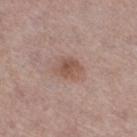Impression: Captured during whole-body skin photography for melanoma surveillance; the lesion was not biopsied. Background: Automated image analysis of the tile measured an area of roughly 6 mm². And it measured a lesion color around L≈52 a*≈19 b*≈25 in CIELAB, a lesion–skin lightness drop of about 9, and a lesion-to-skin contrast of about 7 (normalized; higher = more distinct). It also reported a classifier nevus-likeness of about 40/100. A female subject, about 60 years old. Imaged with white-light lighting. The lesion's longest dimension is about 3 mm. Cropped from a total-body skin-imaging series; the visible field is about 15 mm. From the leg.